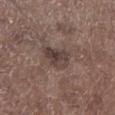Q: Was this lesion biopsied?
A: total-body-photography surveillance lesion; no biopsy
Q: Who is the patient?
A: male, aged 68 to 72
Q: What is the anatomic site?
A: the left lower leg
Q: What lighting was used for the tile?
A: white-light illumination
Q: What is the imaging modality?
A: total-body-photography crop, ~15 mm field of view